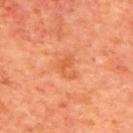Assessment:
Recorded during total-body skin imaging; not selected for excision or biopsy.
Context:
A 15 mm crop from a total-body photograph taken for skin-cancer surveillance. An algorithmic analysis of the crop reported a lesion area of about 3.5 mm², a shape eccentricity near 0.75, and a symmetry-axis asymmetry near 0.55. It also reported an average lesion color of about L≈53 a*≈30 b*≈40 (CIELAB) and a normalized lesion–skin contrast near 5.5. The subject is a male roughly 65 years of age. This is a cross-polarized tile. On the upper back. The lesion's longest dimension is about 2.5 mm.